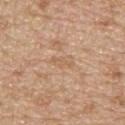Q: Is there a histopathology result?
A: imaged on a skin check; not biopsied
Q: What are the patient's age and sex?
A: male, aged around 70
Q: Lesion location?
A: the back
Q: Automated lesion metrics?
A: a nevus-likeness score of about 0/100 and lesion-presence confidence of about 95/100
Q: How was the tile lit?
A: white-light illumination
Q: Lesion size?
A: about 2.5 mm
Q: What kind of image is this?
A: ~15 mm tile from a whole-body skin photo